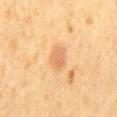Clinical impression: Captured during whole-body skin photography for melanoma surveillance; the lesion was not biopsied.